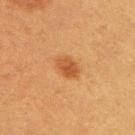Recorded during total-body skin imaging; not selected for excision or biopsy.
Located on the right upper arm.
A region of skin cropped from a whole-body photographic capture, roughly 15 mm wide.
The subject is a female approximately 40 years of age.
Captured under cross-polarized illumination.
About 3.5 mm across.